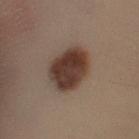Q: What lighting was used for the tile?
A: cross-polarized
Q: What did automated image analysis measure?
A: a lesion color around L≈30 a*≈14 b*≈20 in CIELAB, about 13 CIELAB-L* units darker than the surrounding skin, and a normalized lesion–skin contrast near 12.5; a nevus-likeness score of about 100/100 and a detector confidence of about 100 out of 100 that the crop contains a lesion
Q: What is the anatomic site?
A: the left lower leg
Q: What are the patient's age and sex?
A: female, aged 48 to 52
Q: How large is the lesion?
A: about 5.5 mm
Q: How was this image acquired?
A: ~15 mm tile from a whole-body skin photo